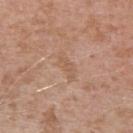<record>
<site>left upper arm</site>
<patient>
  <sex>male</sex>
  <age_approx>40</age_approx>
</patient>
<lighting>white-light</lighting>
<image>
  <source>total-body photography crop</source>
  <field_of_view_mm>15</field_of_view_mm>
</image>
</record>